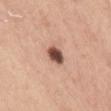This lesion was catalogued during total-body skin photography and was not selected for biopsy.
A female patient, approximately 30 years of age.
Longest diameter approximately 3 mm.
A close-up tile cropped from a whole-body skin photograph, about 15 mm across.
Automated image analysis of the tile measured a footprint of about 5 mm² and an outline eccentricity of about 0.75 (0 = round, 1 = elongated). The analysis additionally found a lesion–skin lightness drop of about 20 and a lesion-to-skin contrast of about 13 (normalized; higher = more distinct). The analysis additionally found an automated nevus-likeness rating near 100 out of 100 and a detector confidence of about 100 out of 100 that the crop contains a lesion.
On the chest.
Imaged with white-light lighting.